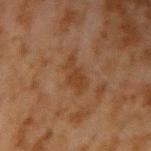The lesion was photographed on a routine skin check and not biopsied; there is no pathology result. The lesion is on the arm. This is a cross-polarized tile. Cropped from a total-body skin-imaging series; the visible field is about 15 mm. A male patient, aged approximately 45. The recorded lesion diameter is about 4 mm.